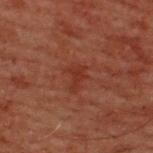This lesion was catalogued during total-body skin photography and was not selected for biopsy. This is a cross-polarized tile. A 15 mm crop from a total-body photograph taken for skin-cancer surveillance. The lesion is located on the upper back. A male patient, about 60 years old. Measured at roughly 3 mm in maximum diameter.